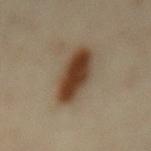biopsy_status: not biopsied; imaged during a skin examination
site: mid back
patient:
  sex: female
  age_approx: 35
lighting: cross-polarized
image:
  source: total-body photography crop
  field_of_view_mm: 15
lesion_size:
  long_diameter_mm_approx: 7.0
automated_metrics:
  cielab_L: 38
  cielab_a: 16
  cielab_b: 28
  vs_skin_darker_L: 16.0
  nevus_likeness_0_100: 100
  lesion_detection_confidence_0_100: 100